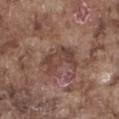workup — imaged on a skin check; not biopsied
image-analysis metrics — a lesion color around L≈42 a*≈18 b*≈23 in CIELAB and a normalized lesion–skin contrast near 7; a border-irregularity index near 5.5/10, internal color variation of about 3 on a 0–10 scale, and a peripheral color-asymmetry measure near 1; lesion-presence confidence of about 100/100
illumination — white-light illumination
subject — male, aged 73 to 77
anatomic site — the right thigh
image — ~15 mm tile from a whole-body skin photo
lesion diameter — about 4.5 mm